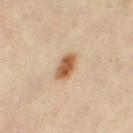Q: Was a biopsy performed?
A: imaged on a skin check; not biopsied
Q: Where on the body is the lesion?
A: the right thigh
Q: What kind of image is this?
A: 15 mm crop, total-body photography
Q: Lesion size?
A: ≈3 mm
Q: Who is the patient?
A: female, approximately 45 years of age